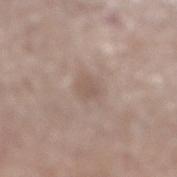  image:
    source: total-body photography crop
    field_of_view_mm: 15
  patient:
    sex: male
    age_approx: 65
  site: right lower leg
  lesion_size:
    long_diameter_mm_approx: 3.5